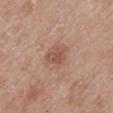Notes:
* workup — no biopsy performed (imaged during a skin exam)
* acquisition — 15 mm crop, total-body photography
* subject — female, aged 63 to 67
* anatomic site — the chest
* image-analysis metrics — an eccentricity of roughly 0.5 and two-axis asymmetry of about 0.3; an average lesion color of about L≈52 a*≈22 b*≈28 (CIELAB), roughly 9 lightness units darker than nearby skin, and a normalized border contrast of about 6.5; a border-irregularity index near 2.5/10, a within-lesion color-variation index near 1.5/10, and a peripheral color-asymmetry measure near 0.5; a classifier nevus-likeness of about 35/100 and a lesion-detection confidence of about 100/100
* tile lighting — white-light
* lesion diameter — ~2.5 mm (longest diameter)